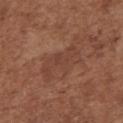Background:
A region of skin cropped from a whole-body photographic capture, roughly 15 mm wide. The lesion-visualizer software estimated about 6 CIELAB-L* units darker than the surrounding skin and a normalized border contrast of about 5. The analysis additionally found a lesion-detection confidence of about 95/100. This is a white-light tile. On the chest. The subject is a female about 75 years old.